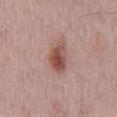Assessment: Recorded during total-body skin imaging; not selected for excision or biopsy. Image and clinical context: The lesion is located on the back. Imaged with white-light lighting. A male patient in their mid- to late 60s. The recorded lesion diameter is about 4.5 mm. A 15 mm close-up tile from a total-body photography series done for melanoma screening. The total-body-photography lesion software estimated a lesion–skin lightness drop of about 12. The analysis additionally found a color-variation rating of about 6/10 and peripheral color asymmetry of about 2. And it measured a nevus-likeness score of about 90/100 and a lesion-detection confidence of about 100/100.